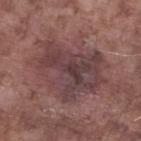Imaged during a routine full-body skin examination; the lesion was not biopsied and no histopathology is available.
A 15 mm close-up tile from a total-body photography series done for melanoma screening.
Located on the leg.
The total-body-photography lesion software estimated an average lesion color of about L≈40 a*≈19 b*≈17 (CIELAB) and a lesion-to-skin contrast of about 7.5 (normalized; higher = more distinct).
The patient is a male roughly 75 years of age.